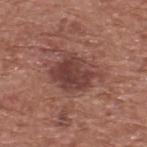Findings:
• workup: total-body-photography surveillance lesion; no biopsy
• body site: the upper back
• image source: total-body-photography crop, ~15 mm field of view
• subject: male, aged 73–77